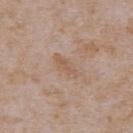{
  "biopsy_status": "not biopsied; imaged during a skin examination",
  "automated_metrics": {
    "cielab_L": 57,
    "cielab_a": 17,
    "cielab_b": 29,
    "vs_skin_contrast_norm": 5.5,
    "nevus_likeness_0_100": 0
  },
  "lesion_size": {
    "long_diameter_mm_approx": 4.0
  },
  "patient": {
    "sex": "male",
    "age_approx": 65
  },
  "image": {
    "source": "total-body photography crop",
    "field_of_view_mm": 15
  },
  "site": "abdomen"
}